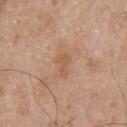<case>
<biopsy_status>not biopsied; imaged during a skin examination</biopsy_status>
<image>
  <source>total-body photography crop</source>
  <field_of_view_mm>15</field_of_view_mm>
</image>
<patient>
  <sex>male</sex>
  <age_approx>65</age_approx>
</patient>
<lighting>white-light</lighting>
<lesion_size>
  <long_diameter_mm_approx>3.0</long_diameter_mm_approx>
</lesion_size>
<site>chest</site>
</case>